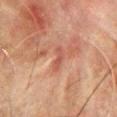The lesion was photographed on a routine skin check and not biopsied; there is no pathology result. This is a cross-polarized tile. Approximately 3 mm at its widest. A male subject, aged 63–67. The lesion is on the front of the torso. A 15 mm close-up extracted from a 3D total-body photography capture.